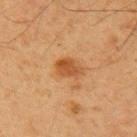{
  "biopsy_status": "not biopsied; imaged during a skin examination",
  "patient": {
    "sex": "male",
    "age_approx": 70
  },
  "lesion_size": {
    "long_diameter_mm_approx": 3.0
  },
  "image": {
    "source": "total-body photography crop",
    "field_of_view_mm": 15
  },
  "lighting": "cross-polarized",
  "site": "arm"
}